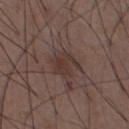Findings:
– notes · catalogued during a skin exam; not biopsied
– image · total-body-photography crop, ~15 mm field of view
– anatomic site · the abdomen
– subject · male, in their 50s